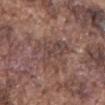The lesion's longest dimension is about 5.5 mm. A roughly 15 mm field-of-view crop from a total-body skin photograph. A male patient, aged approximately 75. From the mid back. Imaged with white-light lighting.